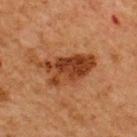Imaged during a routine full-body skin examination; the lesion was not biopsied and no histopathology is available.
This image is a 15 mm lesion crop taken from a total-body photograph.
The subject is a male aged 63 to 67.
The lesion-visualizer software estimated an outline eccentricity of about 0.85 (0 = round, 1 = elongated). And it measured border irregularity of about 4.5 on a 0–10 scale, a within-lesion color-variation index near 5.5/10, and peripheral color asymmetry of about 2. And it measured an automated nevus-likeness rating near 65 out of 100 and a lesion-detection confidence of about 100/100.
Located on the upper back.
Imaged with cross-polarized lighting.